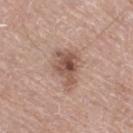workup: no biopsy performed (imaged during a skin exam); site: the right thigh; illumination: white-light; subject: male, in their mid- to late 70s; imaging modality: ~15 mm tile from a whole-body skin photo.